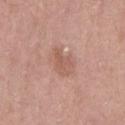Findings:
- notes · no biopsy performed (imaged during a skin exam)
- location · the left thigh
- acquisition · ~15 mm tile from a whole-body skin photo
- patient · female, aged around 40